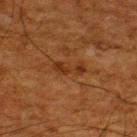Clinical impression: This lesion was catalogued during total-body skin photography and was not selected for biopsy. Context: Located on the upper back. Cropped from a total-body skin-imaging series; the visible field is about 15 mm. The subject is a male aged 63 to 67. The recorded lesion diameter is about 4 mm.